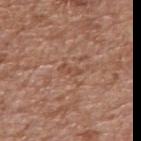Clinical impression: Captured during whole-body skin photography for melanoma surveillance; the lesion was not biopsied. Background: A region of skin cropped from a whole-body photographic capture, roughly 15 mm wide. The lesion is on the right upper arm. The patient is a male in their 70s. About 2.5 mm across. Imaged with white-light lighting. The lesion-visualizer software estimated two-axis asymmetry of about 0.45. It also reported a mean CIELAB color near L≈49 a*≈22 b*≈30 and a normalized lesion–skin contrast near 5.5.